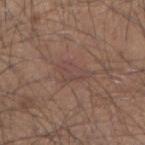– notes — catalogued during a skin exam; not biopsied
– imaging modality — ~15 mm crop, total-body skin-cancer survey
– site — the right forearm
– subject — male, approximately 35 years of age